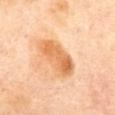notes = total-body-photography surveillance lesion; no biopsy
anatomic site = the mid back
image-analysis metrics = an area of roughly 14 mm² and a shape eccentricity near 0.85
size = ~5.5 mm (longest diameter)
patient = female, about 60 years old
lighting = cross-polarized
image = ~15 mm tile from a whole-body skin photo